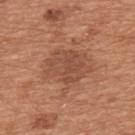Case summary:
• notes: imaged on a skin check; not biopsied
• lesion diameter: ~5.5 mm (longest diameter)
• patient: male, roughly 65 years of age
• lighting: white-light
• site: the upper back
• imaging modality: ~15 mm tile from a whole-body skin photo